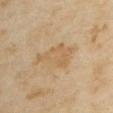Assessment:
The lesion was tiled from a total-body skin photograph and was not biopsied.
Context:
The patient is a female in their mid- to late 30s. Cropped from a whole-body photographic skin survey; the tile spans about 15 mm. Captured under cross-polarized illumination. The lesion-visualizer software estimated an outline eccentricity of about 0.85 (0 = round, 1 = elongated) and a symmetry-axis asymmetry near 0.45. The analysis additionally found a lesion-detection confidence of about 100/100. Located on the upper back.